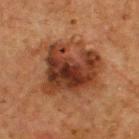follow-up — imaged on a skin check; not biopsied
lighting — cross-polarized
image-analysis metrics — a symmetry-axis asymmetry near 0.3; a classifier nevus-likeness of about 50/100 and lesion-presence confidence of about 100/100
subject — male, approximately 75 years of age
site — the upper back
image — 15 mm crop, total-body photography
lesion diameter — ~8.5 mm (longest diameter)